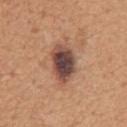notes — imaged on a skin check; not biopsied | subject — male, aged 53 to 57 | lesion diameter — ≈5.5 mm | image-analysis metrics — an average lesion color of about L≈47 a*≈20 b*≈25 (CIELAB), roughly 16 lightness units darker than nearby skin, and a normalized lesion–skin contrast near 12.5 | image source — ~15 mm crop, total-body skin-cancer survey | location — the chest | lighting — white-light.